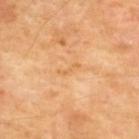biopsy status = total-body-photography surveillance lesion; no biopsy
acquisition = 15 mm crop, total-body photography
location = the upper back
subject = male, roughly 55 years of age